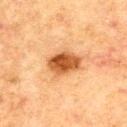Recorded during total-body skin imaging; not selected for excision or biopsy. Automated image analysis of the tile measured a footprint of about 10 mm² and a symmetry-axis asymmetry near 0.2. It also reported a border-irregularity rating of about 2/10, a within-lesion color-variation index near 5.5/10, and peripheral color asymmetry of about 2. And it measured a nevus-likeness score of about 100/100. The subject is a male aged around 70. A 15 mm close-up extracted from a 3D total-body photography capture. From the upper back. Captured under cross-polarized illumination. Longest diameter approximately 4 mm.